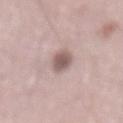notes: no biopsy performed (imaged during a skin exam)
image-analysis metrics: a lesion area of about 6 mm²; a border-irregularity rating of about 2/10, a within-lesion color-variation index near 3/10, and radial color variation of about 1
subject: male, aged approximately 50
lighting: white-light illumination
lesion diameter: ~4 mm (longest diameter)
anatomic site: the mid back
acquisition: total-body-photography crop, ~15 mm field of view